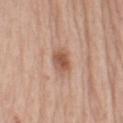notes = imaged on a skin check; not biopsied | location = the arm | image = total-body-photography crop, ~15 mm field of view | tile lighting = white-light illumination | patient = male, in their mid-70s | lesion size = about 3 mm.